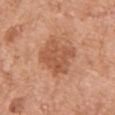workup = catalogued during a skin exam; not biopsied
subject = male, aged 78–82
anatomic site = the chest
image source = total-body-photography crop, ~15 mm field of view